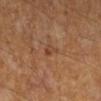Assessment:
Recorded during total-body skin imaging; not selected for excision or biopsy.
Acquisition and patient details:
The subject is a male aged around 60. A close-up tile cropped from a whole-body skin photograph, about 15 mm across. The lesion's longest dimension is about 2.5 mm. The lesion is located on the right lower leg. An algorithmic analysis of the crop reported a lesion area of about 3 mm², a shape eccentricity near 0.85, and a shape-asymmetry score of about 0.45 (0 = symmetric). It also reported a border-irregularity rating of about 4.5/10 and a within-lesion color-variation index near 2.5/10.